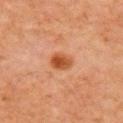Captured during whole-body skin photography for melanoma surveillance; the lesion was not biopsied. Automated image analysis of the tile measured a footprint of about 5.5 mm². The analysis additionally found a lesion color around L≈43 a*≈25 b*≈35 in CIELAB and a normalized lesion–skin contrast near 9. The software also gave a within-lesion color-variation index near 4/10. The analysis additionally found a detector confidence of about 100 out of 100 that the crop contains a lesion. On the chest. A 15 mm close-up extracted from a 3D total-body photography capture. This is a cross-polarized tile. A male subject, aged 68–72. Measured at roughly 2.5 mm in maximum diameter.